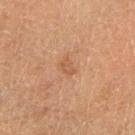No biopsy was performed on this lesion — it was imaged during a full skin examination and was not determined to be concerning. A male subject aged approximately 65. A 15 mm close-up extracted from a 3D total-body photography capture. The lesion is on the right forearm.